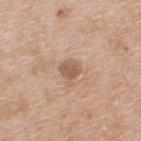Acquisition and patient details: The lesion-visualizer software estimated a mean CIELAB color near L≈57 a*≈17 b*≈31, roughly 10 lightness units darker than nearby skin, and a normalized lesion–skin contrast near 7. It also reported an automated nevus-likeness rating near 0 out of 100 and a detector confidence of about 100 out of 100 that the crop contains a lesion. The patient is a male aged 63–67. Longest diameter approximately 2.5 mm. On the back. A region of skin cropped from a whole-body photographic capture, roughly 15 mm wide.